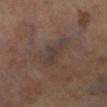workup: imaged on a skin check; not biopsied
patient: male, about 70 years old
site: the left lower leg
acquisition: ~15 mm tile from a whole-body skin photo
illumination: cross-polarized